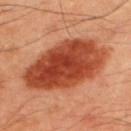Q: Was this lesion biopsied?
A: catalogued during a skin exam; not biopsied
Q: Patient demographics?
A: male, roughly 50 years of age
Q: What kind of image is this?
A: total-body-photography crop, ~15 mm field of view
Q: What lighting was used for the tile?
A: cross-polarized illumination
Q: What is the anatomic site?
A: the upper back